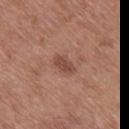| field | value |
|---|---|
| notes | catalogued during a skin exam; not biopsied |
| body site | the mid back |
| subject | male, aged 68 to 72 |
| lighting | white-light illumination |
| image | total-body-photography crop, ~15 mm field of view |